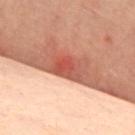Background: Measured at roughly 4 mm in maximum diameter. On the front of the torso. Imaged with cross-polarized lighting. A lesion tile, about 15 mm wide, cut from a 3D total-body photograph. A female patient aged approximately 55.